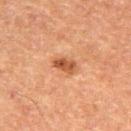Imaged during a routine full-body skin examination; the lesion was not biopsied and no histopathology is available. The lesion is located on the right thigh. Automated image analysis of the tile measured a lesion color around L≈43 a*≈23 b*≈33 in CIELAB and a normalized lesion–skin contrast near 9. It also reported a within-lesion color-variation index near 3.5/10 and a peripheral color-asymmetry measure near 1.5. It also reported an automated nevus-likeness rating near 80 out of 100 and lesion-presence confidence of about 100/100. Measured at roughly 2.5 mm in maximum diameter. A 15 mm close-up tile from a total-body photography series done for melanoma screening. Captured under cross-polarized illumination. The patient is a female aged approximately 60.